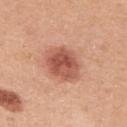The lesion was tiled from a total-body skin photograph and was not biopsied. From the right upper arm. Cropped from a whole-body photographic skin survey; the tile spans about 15 mm. The tile uses white-light illumination. A female patient, in their mid-40s. Approximately 4.5 mm at its widest.